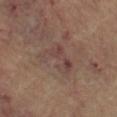workup — no biopsy performed (imaged during a skin exam)
subject — female, in their mid- to late 60s
illumination — cross-polarized
anatomic site — the left thigh
acquisition — ~15 mm crop, total-body skin-cancer survey
lesion diameter — ~5.5 mm (longest diameter)